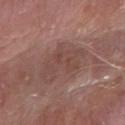biopsy_status: not biopsied; imaged during a skin examination
lesion_size:
  long_diameter_mm_approx: 7.0
patient:
  sex: male
  age_approx: 65
image:
  source: total-body photography crop
  field_of_view_mm: 15
site: arm
lighting: white-light